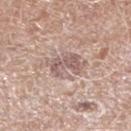No biopsy was performed on this lesion — it was imaged during a full skin examination and was not determined to be concerning. A male subject aged around 80. The total-body-photography lesion software estimated a lesion color around L≈58 a*≈17 b*≈21 in CIELAB, roughly 10 lightness units darker than nearby skin, and a normalized border contrast of about 6.5. And it measured a border-irregularity index near 5.5/10, a within-lesion color-variation index near 3.5/10, and radial color variation of about 1. Located on the left lower leg. Captured under white-light illumination. A roughly 15 mm field-of-view crop from a total-body skin photograph.